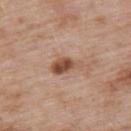Imaged during a routine full-body skin examination; the lesion was not biopsied and no histopathology is available. The subject is a male aged 53 to 57. A region of skin cropped from a whole-body photographic capture, roughly 15 mm wide. Automated image analysis of the tile measured a border-irregularity index near 3.5/10, internal color variation of about 4.5 on a 0–10 scale, and a peripheral color-asymmetry measure near 1.5. The software also gave an automated nevus-likeness rating near 90 out of 100 and lesion-presence confidence of about 100/100. The lesion's longest dimension is about 4 mm. On the upper back. Captured under white-light illumination.